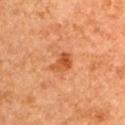Recorded during total-body skin imaging; not selected for excision or biopsy. A lesion tile, about 15 mm wide, cut from a 3D total-body photograph. The lesion is on the back. A female patient, roughly 60 years of age. Imaged with cross-polarized lighting. Measured at roughly 3 mm in maximum diameter.